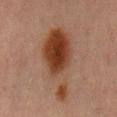Part of a total-body skin-imaging series; this lesion was reviewed on a skin check and was not flagged for biopsy. Located on the abdomen. The tile uses cross-polarized illumination. The lesion-visualizer software estimated a lesion-detection confidence of about 100/100. A male subject about 70 years old. A 15 mm close-up tile from a total-body photography series done for melanoma screening.